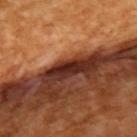{
  "patient": {
    "sex": "female",
    "age_approx": 55
  },
  "site": "left upper arm",
  "lighting": "cross-polarized",
  "image": {
    "source": "total-body photography crop",
    "field_of_view_mm": 15
  }
}